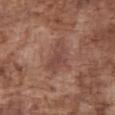Part of a total-body skin-imaging series; this lesion was reviewed on a skin check and was not flagged for biopsy.
The lesion-visualizer software estimated an area of roughly 8 mm² and a shape eccentricity near 0.8. It also reported an average lesion color of about L≈45 a*≈22 b*≈24 (CIELAB) and a normalized border contrast of about 6.
Approximately 4 mm at its widest.
A lesion tile, about 15 mm wide, cut from a 3D total-body photograph.
From the abdomen.
A male subject roughly 75 years of age.